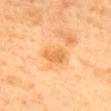<case>
<lighting>cross-polarized</lighting>
<automated_metrics>
  <area_mm2_approx>5.0</area_mm2_approx>
  <eccentricity>0.75</eccentricity>
  <shape_asymmetry>0.3</shape_asymmetry>
  <cielab_L>60</cielab_L>
  <cielab_a>23</cielab_a>
  <cielab_b>44</cielab_b>
  <vs_skin_darker_L>8.0</vs_skin_darker_L>
  <vs_skin_contrast_norm>6.5</vs_skin_contrast_norm>
  <border_irregularity_0_10>3.0</border_irregularity_0_10>
  <color_variation_0_10>1.5</color_variation_0_10>
  <nevus_likeness_0_100>0</nevus_likeness_0_100>
  <lesion_detection_confidence_0_100>100</lesion_detection_confidence_0_100>
</automated_metrics>
<lesion_size>
  <long_diameter_mm_approx>3.0</long_diameter_mm_approx>
</lesion_size>
<patient>
  <sex>female</sex>
  <age_approx>60</age_approx>
</patient>
<site>back</site>
<image>
  <source>total-body photography crop</source>
  <field_of_view_mm>15</field_of_view_mm>
</image>
</case>